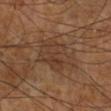{
  "biopsy_status": "not biopsied; imaged during a skin examination",
  "lesion_size": {
    "long_diameter_mm_approx": 5.5
  },
  "lighting": "cross-polarized",
  "image": {
    "source": "total-body photography crop",
    "field_of_view_mm": 15
  },
  "site": "right lower leg",
  "patient": {
    "sex": "male",
    "age_approx": 70
  }
}